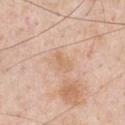Impression:
Captured during whole-body skin photography for melanoma surveillance; the lesion was not biopsied.
Image and clinical context:
A close-up tile cropped from a whole-body skin photograph, about 15 mm across. Imaged with white-light lighting. A male subject roughly 50 years of age. On the chest. Automated tile analysis of the lesion measured a nevus-likeness score of about 0/100 and a detector confidence of about 100 out of 100 that the crop contains a lesion. Measured at roughly 2.5 mm in maximum diameter.